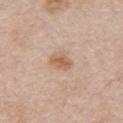* follow-up · imaged on a skin check; not biopsied
* diameter · ~3 mm (longest diameter)
* imaging modality · ~15 mm tile from a whole-body skin photo
* illumination · white-light
* subject · female, approximately 65 years of age
* image-analysis metrics · a mean CIELAB color near L≈61 a*≈18 b*≈33, a lesion–skin lightness drop of about 9, and a lesion-to-skin contrast of about 7.5 (normalized; higher = more distinct)
* body site · the chest